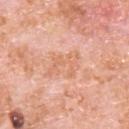<case>
<biopsy_status>not biopsied; imaged during a skin examination</biopsy_status>
<image>
  <source>total-body photography crop</source>
  <field_of_view_mm>15</field_of_view_mm>
</image>
<patient>
  <sex>male</sex>
  <age_approx>80</age_approx>
</patient>
<site>upper back</site>
</case>